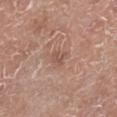The subject is a male aged 78–82.
Captured under white-light illumination.
The lesion is located on the right lower leg.
The lesion's longest dimension is about 2.5 mm.
Cropped from a whole-body photographic skin survey; the tile spans about 15 mm.